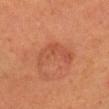Located on the head or neck. A lesion tile, about 15 mm wide, cut from a 3D total-body photograph. The tile uses cross-polarized illumination. A female subject in their 50s.